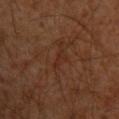Q: Is there a histopathology result?
A: catalogued during a skin exam; not biopsied
Q: How large is the lesion?
A: ≈2.5 mm
Q: How was the tile lit?
A: cross-polarized illumination
Q: How was this image acquired?
A: ~15 mm tile from a whole-body skin photo
Q: What is the anatomic site?
A: the chest
Q: What did automated image analysis measure?
A: an average lesion color of about L≈27 a*≈21 b*≈26 (CIELAB), roughly 4 lightness units darker than nearby skin, and a normalized lesion–skin contrast near 5
Q: Patient demographics?
A: male, roughly 30 years of age